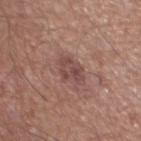Clinical impression: The lesion was tiled from a total-body skin photograph and was not biopsied. Image and clinical context: The subject is a male aged approximately 65. From the chest. Automated tile analysis of the lesion measured a shape eccentricity near 0.7 and two-axis asymmetry of about 0.35. The analysis additionally found internal color variation of about 4 on a 0–10 scale and peripheral color asymmetry of about 1.5. Imaged with white-light lighting. The recorded lesion diameter is about 3.5 mm. A region of skin cropped from a whole-body photographic capture, roughly 15 mm wide.